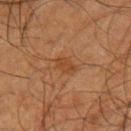Assessment:
Part of a total-body skin-imaging series; this lesion was reviewed on a skin check and was not flagged for biopsy.
Clinical summary:
The total-body-photography lesion software estimated an outline eccentricity of about 0.8 (0 = round, 1 = elongated) and two-axis asymmetry of about 0.25. The software also gave a mean CIELAB color near L≈36 a*≈19 b*≈31, about 6 CIELAB-L* units darker than the surrounding skin, and a normalized lesion–skin contrast near 6. The lesion is located on the left upper arm. This is a cross-polarized tile. A roughly 15 mm field-of-view crop from a total-body skin photograph. Measured at roughly 3 mm in maximum diameter. A male patient aged approximately 65.